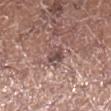{
  "biopsy_status": "not biopsied; imaged during a skin examination",
  "patient": {
    "sex": "female",
    "age_approx": 50
  },
  "lighting": "white-light",
  "site": "leg",
  "image": {
    "source": "total-body photography crop",
    "field_of_view_mm": 15
  }
}